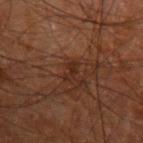<case>
  <biopsy_status>not biopsied; imaged during a skin examination</biopsy_status>
  <lighting>cross-polarized</lighting>
  <patient>
    <sex>male</sex>
    <age_approx>70</age_approx>
  </patient>
  <image>
    <source>total-body photography crop</source>
    <field_of_view_mm>15</field_of_view_mm>
  </image>
  <site>arm</site>
  <lesion_size>
    <long_diameter_mm_approx>2.5</long_diameter_mm_approx>
  </lesion_size>
</case>